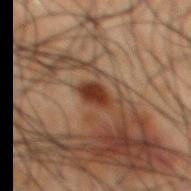The patient is a male in their 50s.
About 3 mm across.
On the back.
A 15 mm close-up extracted from a 3D total-body photography capture.
This is a cross-polarized tile.